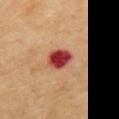Recorded during total-body skin imaging; not selected for excision or biopsy. Approximately 3 mm at its widest. Cropped from a whole-body photographic skin survey; the tile spans about 15 mm. Captured under cross-polarized illumination. The subject is a male aged around 70. Located on the mid back.